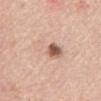The lesion was tiled from a total-body skin photograph and was not biopsied. Cropped from a whole-body photographic skin survey; the tile spans about 15 mm. The subject is a female about 65 years old. Located on the mid back.